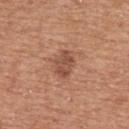The lesion was photographed on a routine skin check and not biopsied; there is no pathology result. A female subject, aged 58 to 62. From the upper back. An algorithmic analysis of the crop reported a footprint of about 5.5 mm², a shape eccentricity near 0.75, and a shape-asymmetry score of about 0.25 (0 = symmetric). The analysis additionally found a lesion–skin lightness drop of about 10 and a normalized border contrast of about 7. It also reported a nevus-likeness score of about 10/100. Imaged with white-light lighting. A lesion tile, about 15 mm wide, cut from a 3D total-body photograph.